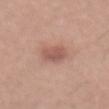Q: Was this lesion biopsied?
A: no biopsy performed (imaged during a skin exam)
Q: What is the lesion's diameter?
A: about 3.5 mm
Q: What kind of image is this?
A: ~15 mm crop, total-body skin-cancer survey
Q: What is the anatomic site?
A: the mid back
Q: What are the patient's age and sex?
A: male, in their mid- to late 40s
Q: Illumination type?
A: white-light